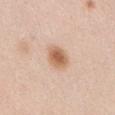Imaged during a routine full-body skin examination; the lesion was not biopsied and no histopathology is available. Measured at roughly 3 mm in maximum diameter. A female subject roughly 40 years of age. This is a white-light tile. The lesion is on the abdomen. This image is a 15 mm lesion crop taken from a total-body photograph.